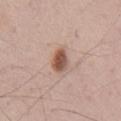Impression: The lesion was photographed on a routine skin check and not biopsied; there is no pathology result. Clinical summary: Automated image analysis of the tile measured a nevus-likeness score of about 95/100. This is a white-light tile. From the chest. A male subject, aged 68–72. A 15 mm close-up extracted from a 3D total-body photography capture.